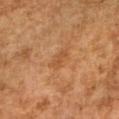follow-up=total-body-photography surveillance lesion; no biopsy | subject=female, in their 60s | image-analysis metrics=a mean CIELAB color near L≈45 a*≈21 b*≈34, roughly 6 lightness units darker than nearby skin, and a normalized lesion–skin contrast near 5; a border-irregularity rating of about 2.5/10, internal color variation of about 1.5 on a 0–10 scale, and peripheral color asymmetry of about 0.5; a nevus-likeness score of about 0/100 and lesion-presence confidence of about 100/100 | image=total-body-photography crop, ~15 mm field of view | anatomic site=the right upper arm | size=≈3 mm.